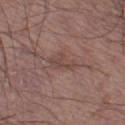The lesion was tiled from a total-body skin photograph and was not biopsied. On the left thigh. A close-up tile cropped from a whole-body skin photograph, about 15 mm across. The lesion-visualizer software estimated an outline eccentricity of about 0.9 (0 = round, 1 = elongated) and two-axis asymmetry of about 0.35. The software also gave border irregularity of about 4.5 on a 0–10 scale, a color-variation rating of about 1/10, and radial color variation of about 0.5. It also reported a lesion-detection confidence of about 80/100. The lesion's longest dimension is about 4 mm. A male patient aged around 55.